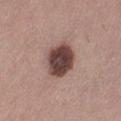Clinical impression: No biopsy was performed on this lesion — it was imaged during a full skin examination and was not determined to be concerning. Acquisition and patient details: The total-body-photography lesion software estimated a lesion color around L≈42 a*≈19 b*≈20 in CIELAB, roughly 19 lightness units darker than nearby skin, and a normalized border contrast of about 13.5. The software also gave a color-variation rating of about 4.5/10 and a peripheral color-asymmetry measure near 1. It also reported a nevus-likeness score of about 35/100 and a detector confidence of about 100 out of 100 that the crop contains a lesion. A female patient, approximately 55 years of age. Captured under white-light illumination. Approximately 4.5 mm at its widest. On the leg. Cropped from a whole-body photographic skin survey; the tile spans about 15 mm.